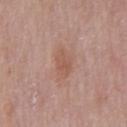Assessment: This lesion was catalogued during total-body skin photography and was not selected for biopsy. Background: Captured under white-light illumination. An algorithmic analysis of the crop reported an outline eccentricity of about 0.8 (0 = round, 1 = elongated) and two-axis asymmetry of about 0.2. The software also gave an average lesion color of about L≈55 a*≈21 b*≈27 (CIELAB), about 7 CIELAB-L* units darker than the surrounding skin, and a normalized border contrast of about 5.5. And it measured border irregularity of about 2 on a 0–10 scale, a color-variation rating of about 2.5/10, and peripheral color asymmetry of about 0.5. The software also gave a nevus-likeness score of about 0/100. A region of skin cropped from a whole-body photographic capture, roughly 15 mm wide. The patient is a male approximately 75 years of age. Longest diameter approximately 3.5 mm. From the mid back.